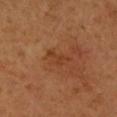Captured during whole-body skin photography for melanoma surveillance; the lesion was not biopsied. Automated image analysis of the tile measured a shape eccentricity near 0.85 and a symmetry-axis asymmetry near 0.45. The software also gave a within-lesion color-variation index near 1/10 and peripheral color asymmetry of about 0.5. Approximately 3.5 mm at its widest. A close-up tile cropped from a whole-body skin photograph, about 15 mm across. The tile uses cross-polarized illumination. The patient is a female approximately 65 years of age. From the left forearm.